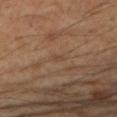Image and clinical context: Imaged with cross-polarized lighting. Automated tile analysis of the lesion measured an outline eccentricity of about 0.85 (0 = round, 1 = elongated). It also reported lesion-presence confidence of about 90/100. Measured at roughly 2 mm in maximum diameter. A female subject aged approximately 60. From the left forearm. A 15 mm close-up tile from a total-body photography series done for melanoma screening.